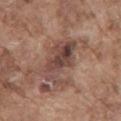biopsy status: total-body-photography surveillance lesion; no biopsy | image source: 15 mm crop, total-body photography | image-analysis metrics: an automated nevus-likeness rating near 0 out of 100 | subject: male, aged around 75 | diameter: ~6 mm (longest diameter) | body site: the mid back.